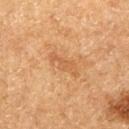Q: Was this lesion biopsied?
A: catalogued during a skin exam; not biopsied
Q: Lesion location?
A: the left thigh
Q: What are the patient's age and sex?
A: male, aged 73–77
Q: What kind of image is this?
A: ~15 mm tile from a whole-body skin photo
Q: Illumination type?
A: cross-polarized illumination